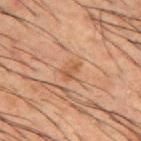Assessment:
No biopsy was performed on this lesion — it was imaged during a full skin examination and was not determined to be concerning.
Clinical summary:
Located on the mid back. Imaged with cross-polarized lighting. A male patient, aged 48 to 52. Approximately 2.5 mm at its widest. Cropped from a total-body skin-imaging series; the visible field is about 15 mm.